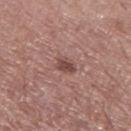Clinical impression:
The lesion was photographed on a routine skin check and not biopsied; there is no pathology result.
Background:
From the left thigh. A 15 mm close-up extracted from a 3D total-body photography capture. An algorithmic analysis of the crop reported a lesion area of about 4 mm², a shape eccentricity near 0.7, and two-axis asymmetry of about 0.2. And it measured a lesion-to-skin contrast of about 7.5 (normalized; higher = more distinct). And it measured a border-irregularity index near 1.5/10, a within-lesion color-variation index near 2/10, and peripheral color asymmetry of about 0.5. The patient is a female in their mid- to late 70s. The tile uses white-light illumination. The lesion's longest dimension is about 2.5 mm.